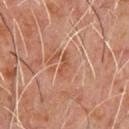Impression: The lesion was tiled from a total-body skin photograph and was not biopsied. Acquisition and patient details: A male patient aged approximately 60. This is a cross-polarized tile. The lesion is located on the chest. Longest diameter approximately 3 mm. A 15 mm close-up extracted from a 3D total-body photography capture.